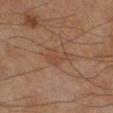Context:
A male patient, in their mid-60s. Cropped from a total-body skin-imaging series; the visible field is about 15 mm. The lesion-visualizer software estimated a lesion area of about 5 mm², an eccentricity of roughly 0.75, and two-axis asymmetry of about 0.35. And it measured about 4 CIELAB-L* units darker than the surrounding skin and a lesion-to-skin contrast of about 4.5 (normalized; higher = more distinct). It also reported a classifier nevus-likeness of about 0/100 and a detector confidence of about 100 out of 100 that the crop contains a lesion. Longest diameter approximately 3 mm. On the leg.